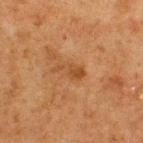Case summary:
• workup — imaged on a skin check; not biopsied
• image source — ~15 mm crop, total-body skin-cancer survey
• automated metrics — an outline eccentricity of about 0.9 (0 = round, 1 = elongated) and a shape-asymmetry score of about 0.55 (0 = symmetric); a border-irregularity index near 6/10, internal color variation of about 1.5 on a 0–10 scale, and a peripheral color-asymmetry measure near 0.5; lesion-presence confidence of about 100/100
• patient — male, approximately 75 years of age
• lesion diameter — about 3.5 mm
• location — the upper back